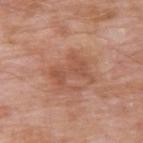Q: Was this lesion biopsied?
A: no biopsy performed (imaged during a skin exam)
Q: What are the patient's age and sex?
A: male, aged 58 to 62
Q: Automated lesion metrics?
A: about 8 CIELAB-L* units darker than the surrounding skin; radial color variation of about 0.5; an automated nevus-likeness rating near 0 out of 100 and a lesion-detection confidence of about 100/100
Q: How was this image acquired?
A: 15 mm crop, total-body photography
Q: How was the tile lit?
A: white-light
Q: Lesion location?
A: the upper back
Q: How large is the lesion?
A: ~4.5 mm (longest diameter)